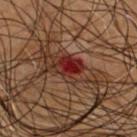This lesion was catalogued during total-body skin photography and was not selected for biopsy.
The patient is a male aged approximately 50.
A 15 mm close-up tile from a total-body photography series done for melanoma screening.
The lesion's longest dimension is about 8 mm.
The lesion is on the upper back.
Imaged with cross-polarized lighting.
The lesion-visualizer software estimated a mean CIELAB color near L≈23 a*≈19 b*≈21, a lesion–skin lightness drop of about 8, and a normalized lesion–skin contrast near 9.5. It also reported a nevus-likeness score of about 0/100 and a lesion-detection confidence of about 100/100.